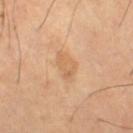| feature | finding |
|---|---|
| follow-up | catalogued during a skin exam; not biopsied |
| subject | male, in their mid- to late 60s |
| image | ~15 mm crop, total-body skin-cancer survey |
| lighting | cross-polarized illumination |
| site | the right thigh |
| lesion size | ≈3.5 mm |